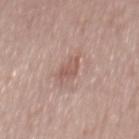Recorded during total-body skin imaging; not selected for excision or biopsy. The lesion's longest dimension is about 3 mm. Cropped from a whole-body photographic skin survey; the tile spans about 15 mm. Automated image analysis of the tile measured a shape eccentricity near 0.85 and a symmetry-axis asymmetry near 0.35. Captured under white-light illumination. From the mid back. The patient is a male about 60 years old.